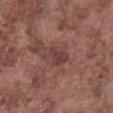Notes:
• notes · total-body-photography surveillance lesion; no biopsy
• acquisition · ~15 mm crop, total-body skin-cancer survey
• patient · male, aged 73–77
• image-analysis metrics · an average lesion color of about L≈40 a*≈23 b*≈21 (CIELAB); a border-irregularity rating of about 3.5/10, internal color variation of about 3 on a 0–10 scale, and peripheral color asymmetry of about 1
• site · the abdomen
• tile lighting · white-light